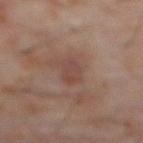Q: Is there a histopathology result?
A: total-body-photography surveillance lesion; no biopsy
Q: Lesion location?
A: the abdomen
Q: Patient demographics?
A: male, aged approximately 60
Q: Illumination type?
A: cross-polarized illumination
Q: How was this image acquired?
A: ~15 mm tile from a whole-body skin photo
Q: What is the lesion's diameter?
A: ≈3.5 mm